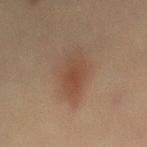No biopsy was performed on this lesion — it was imaged during a full skin examination and was not determined to be concerning.
The lesion is located on the mid back.
A male patient approximately 60 years of age.
A roughly 15 mm field-of-view crop from a total-body skin photograph.
Captured under cross-polarized illumination.
Longest diameter approximately 6.5 mm.